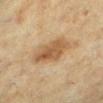This lesion was catalogued during total-body skin photography and was not selected for biopsy. A female patient, in their mid-50s. The tile uses cross-polarized illumination. This image is a 15 mm lesion crop taken from a total-body photograph. The lesion is located on the left lower leg. Approximately 4.5 mm at its widest. An algorithmic analysis of the crop reported a lesion area of about 12 mm², a shape eccentricity near 0.75, and two-axis asymmetry of about 0.2. And it measured an average lesion color of about L≈46 a*≈16 b*≈31 (CIELAB), roughly 10 lightness units darker than nearby skin, and a normalized lesion–skin contrast near 7.5. The software also gave internal color variation of about 4 on a 0–10 scale and radial color variation of about 1.5. The software also gave a nevus-likeness score of about 90/100 and lesion-presence confidence of about 100/100.